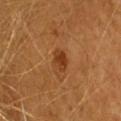Located on the upper back.
This is a cross-polarized tile.
Automated image analysis of the tile measured a mean CIELAB color near L≈38 a*≈25 b*≈38, a lesion–skin lightness drop of about 9, and a normalized lesion–skin contrast near 8. And it measured a nevus-likeness score of about 90/100.
A female patient, approximately 65 years of age.
A 15 mm close-up extracted from a 3D total-body photography capture.